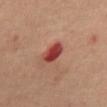Impression:
This lesion was catalogued during total-body skin photography and was not selected for biopsy.
Clinical summary:
The lesion is located on the back. Automated tile analysis of the lesion measured a footprint of about 5.5 mm², an outline eccentricity of about 0.65 (0 = round, 1 = elongated), and two-axis asymmetry of about 0.15. The analysis additionally found an average lesion color of about L≈41 a*≈33 b*≈28 (CIELAB) and a lesion–skin lightness drop of about 14. The software also gave a border-irregularity index near 1.5/10 and peripheral color asymmetry of about 1. It also reported a nevus-likeness score of about 0/100. A male subject aged 68 to 72. A 15 mm crop from a total-body photograph taken for skin-cancer surveillance.